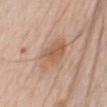{
  "biopsy_status": "not biopsied; imaged during a skin examination",
  "image": {
    "source": "total-body photography crop",
    "field_of_view_mm": 15
  },
  "site": "chest",
  "lighting": "white-light",
  "patient": {
    "sex": "male",
    "age_approx": 80
  },
  "automated_metrics": {
    "area_mm2_approx": 11.0,
    "cielab_L": 59,
    "cielab_a": 20,
    "cielab_b": 32,
    "vs_skin_contrast_norm": 6.5
  }
}